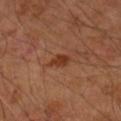Q: Was this lesion biopsied?
A: imaged on a skin check; not biopsied
Q: How was the tile lit?
A: cross-polarized
Q: Automated lesion metrics?
A: an outline eccentricity of about 0.85 (0 = round, 1 = elongated) and two-axis asymmetry of about 0.35; an average lesion color of about L≈35 a*≈24 b*≈31 (CIELAB) and about 9 CIELAB-L* units darker than the surrounding skin; a border-irregularity index near 3/10 and peripheral color asymmetry of about 0.5
Q: Who is the patient?
A: male, aged 63–67
Q: What is the imaging modality?
A: ~15 mm crop, total-body skin-cancer survey
Q: Lesion location?
A: the arm
Q: Lesion size?
A: ≈2.5 mm